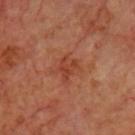The lesion was photographed on a routine skin check and not biopsied; there is no pathology result. Imaged with cross-polarized lighting. This image is a 15 mm lesion crop taken from a total-body photograph. The lesion is located on the chest. The patient is a male aged approximately 70. Automated image analysis of the tile measured a lesion-detection confidence of about 100/100.